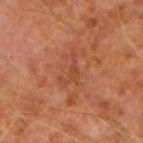Impression: The lesion was photographed on a routine skin check and not biopsied; there is no pathology result. Image and clinical context: From the arm. The subject is a male aged around 60. Cropped from a total-body skin-imaging series; the visible field is about 15 mm. Imaged with cross-polarized lighting. An algorithmic analysis of the crop reported an eccentricity of roughly 0.9. And it measured border irregularity of about 9 on a 0–10 scale, a color-variation rating of about 1/10, and radial color variation of about 0.5. The recorded lesion diameter is about 4 mm.